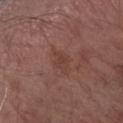No biopsy was performed on this lesion — it was imaged during a full skin examination and was not determined to be concerning.
The lesion is on the right forearm.
A male subject in their mid- to late 70s.
Cropped from a total-body skin-imaging series; the visible field is about 15 mm.